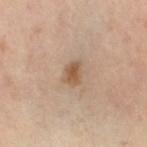Cropped from a whole-body photographic skin survey; the tile spans about 15 mm. A female patient roughly 40 years of age. Located on the left lower leg. Imaged with cross-polarized lighting.